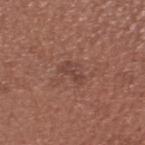biopsy status = catalogued during a skin exam; not biopsied
acquisition = ~15 mm crop, total-body skin-cancer survey
subject = male, roughly 35 years of age
body site = the head or neck
lighting = white-light
size = ~3 mm (longest diameter)